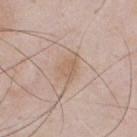{
  "biopsy_status": "not biopsied; imaged during a skin examination",
  "site": "chest",
  "image": {
    "source": "total-body photography crop",
    "field_of_view_mm": 15
  },
  "patient": {
    "sex": "male",
    "age_approx": 55
  }
}